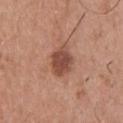workup: imaged on a skin check; not biopsied | body site: the upper back | acquisition: ~15 mm crop, total-body skin-cancer survey | subject: male, in their 50s.